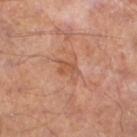notes: no biopsy performed (imaged during a skin exam) | location: the left lower leg | image source: ~15 mm tile from a whole-body skin photo | subject: male, aged approximately 65.